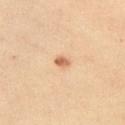biopsy status: total-body-photography surveillance lesion; no biopsy | acquisition: 15 mm crop, total-body photography | anatomic site: the chest | diameter: ~2 mm (longest diameter) | illumination: cross-polarized | subject: female, approximately 40 years of age | automated metrics: a footprint of about 2.5 mm², an eccentricity of roughly 0.75, and two-axis asymmetry of about 0.35; border irregularity of about 3 on a 0–10 scale, a within-lesion color-variation index near 3/10, and a peripheral color-asymmetry measure near 1.5; a detector confidence of about 100 out of 100 that the crop contains a lesion.